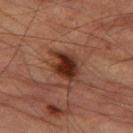The lesion is on the left thigh.
A lesion tile, about 15 mm wide, cut from a 3D total-body photograph.
The patient is a male in their mid-80s or older.